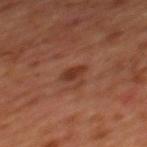Impression:
No biopsy was performed on this lesion — it was imaged during a full skin examination and was not determined to be concerning.
Image and clinical context:
The subject is a male aged around 65. From the mid back. This image is a 15 mm lesion crop taken from a total-body photograph. The recorded lesion diameter is about 2.5 mm. The tile uses cross-polarized illumination.